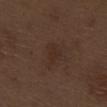| field | value |
|---|---|
| workup | imaged on a skin check; not biopsied |
| subject | male, aged around 70 |
| tile lighting | white-light illumination |
| imaging modality | ~15 mm tile from a whole-body skin photo |
| anatomic site | the right thigh |